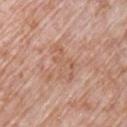{
  "biopsy_status": "not biopsied; imaged during a skin examination",
  "image": {
    "source": "total-body photography crop",
    "field_of_view_mm": 15
  },
  "lighting": "white-light",
  "site": "chest",
  "patient": {
    "sex": "male",
    "age_approx": 70
  }
}